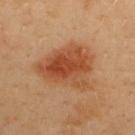Part of a total-body skin-imaging series; this lesion was reviewed on a skin check and was not flagged for biopsy.
The lesion is on the upper back.
A 15 mm crop from a total-body photograph taken for skin-cancer surveillance.
The patient is a male approximately 40 years of age.
Imaged with cross-polarized lighting.
Approximately 7 mm at its widest.
Automated tile analysis of the lesion measured a lesion area of about 24 mm², an outline eccentricity of about 0.6 (0 = round, 1 = elongated), and two-axis asymmetry of about 0.3. It also reported a lesion color around L≈49 a*≈26 b*≈37 in CIELAB, about 11 CIELAB-L* units darker than the surrounding skin, and a normalized lesion–skin contrast near 8.5. The software also gave a border-irregularity rating of about 3.5/10, internal color variation of about 6.5 on a 0–10 scale, and radial color variation of about 2.